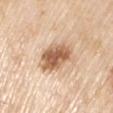{
  "biopsy_status": "not biopsied; imaged during a skin examination"
}